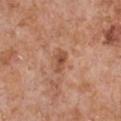{"biopsy_status": "not biopsied; imaged during a skin examination", "lesion_size": {"long_diameter_mm_approx": 2.5}, "site": "chest", "image": {"source": "total-body photography crop", "field_of_view_mm": 15}, "patient": {"sex": "male", "age_approx": 65}, "lighting": "white-light"}